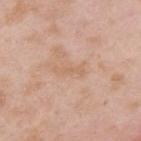The lesion was photographed on a routine skin check and not biopsied; there is no pathology result.
Located on the upper back.
Imaged with white-light lighting.
The lesion's longest dimension is about 4 mm.
A male subject, aged 53–57.
A 15 mm close-up tile from a total-body photography series done for melanoma screening.
Automated tile analysis of the lesion measured an automated nevus-likeness rating near 0 out of 100 and a detector confidence of about 100 out of 100 that the crop contains a lesion.